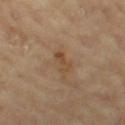This lesion was catalogued during total-body skin photography and was not selected for biopsy. This is a cross-polarized tile. The lesion is located on the leg. Cropped from a total-body skin-imaging series; the visible field is about 15 mm. The subject is a female aged approximately 70.